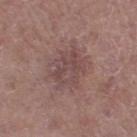{
  "biopsy_status": "not biopsied; imaged during a skin examination",
  "automated_metrics": {
    "border_irregularity_0_10": 4.5,
    "nevus_likeness_0_100": 0,
    "lesion_detection_confidence_0_100": 100
  },
  "patient": {
    "sex": "female",
    "age_approx": 50
  },
  "image": {
    "source": "total-body photography crop",
    "field_of_view_mm": 15
  },
  "lesion_size": {
    "long_diameter_mm_approx": 6.0
  },
  "site": "right thigh",
  "lighting": "white-light"
}